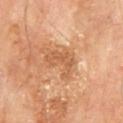Clinical impression: Imaged during a routine full-body skin examination; the lesion was not biopsied and no histopathology is available. Context: The recorded lesion diameter is about 4 mm. The lesion is located on the mid back. A male subject aged approximately 70. A close-up tile cropped from a whole-body skin photograph, about 15 mm across. This is a cross-polarized tile.